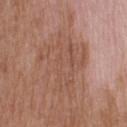{
  "biopsy_status": "not biopsied; imaged during a skin examination",
  "lesion_size": {
    "long_diameter_mm_approx": 7.5
  },
  "automated_metrics": {
    "area_mm2_approx": 23.0,
    "shape_asymmetry": 0.55,
    "cielab_L": 52,
    "cielab_a": 21,
    "cielab_b": 28,
    "vs_skin_contrast_norm": 4.5,
    "border_irregularity_0_10": 8.0,
    "color_variation_0_10": 3.5,
    "peripheral_color_asymmetry": 1.0,
    "lesion_detection_confidence_0_100": 100
  },
  "site": "head or neck",
  "lighting": "white-light",
  "image": {
    "source": "total-body photography crop",
    "field_of_view_mm": 15
  },
  "patient": {
    "sex": "male",
    "age_approx": 70
  }
}